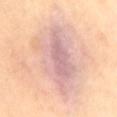notes: total-body-photography surveillance lesion; no biopsy | lesion diameter: ≈11 mm | anatomic site: the mid back | subject: female, roughly 40 years of age | acquisition: 15 mm crop, total-body photography.